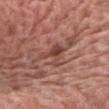Clinical summary: The lesion's longest dimension is about 3.5 mm. A male patient roughly 75 years of age. A region of skin cropped from a whole-body photographic capture, roughly 15 mm wide. From the chest.